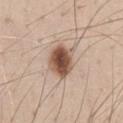Assessment: This lesion was catalogued during total-body skin photography and was not selected for biopsy. Image and clinical context: An algorithmic analysis of the crop reported an area of roughly 10 mm², an outline eccentricity of about 0.6 (0 = round, 1 = elongated), and a shape-asymmetry score of about 0.15 (0 = symmetric). And it measured a mean CIELAB color near L≈52 a*≈19 b*≈28, roughly 18 lightness units darker than nearby skin, and a lesion-to-skin contrast of about 11.5 (normalized; higher = more distinct). The software also gave border irregularity of about 1.5 on a 0–10 scale, a within-lesion color-variation index near 6/10, and peripheral color asymmetry of about 2. The analysis additionally found a nevus-likeness score of about 100/100. A male subject, aged around 45. The tile uses white-light illumination. Longest diameter approximately 4 mm. Located on the abdomen. Cropped from a total-body skin-imaging series; the visible field is about 15 mm.